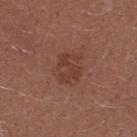Case summary:
* follow-up · imaged on a skin check; not biopsied
* site · the upper back
* illumination · white-light
* image · ~15 mm tile from a whole-body skin photo
* patient · female, aged 23–27
* lesion size · ≈3.5 mm